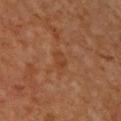biopsy_status: not biopsied; imaged during a skin examination
image:
  source: total-body photography crop
  field_of_view_mm: 15
patient:
  sex: male
  age_approx: 60
automated_metrics:
  border_irregularity_0_10: 2.5
  peripheral_color_asymmetry: 1.0
  nevus_likeness_0_100: 0
  lesion_detection_confidence_0_100: 100
lighting: cross-polarized
lesion_size:
  long_diameter_mm_approx: 2.5
site: upper back